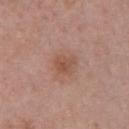Part of a total-body skin-imaging series; this lesion was reviewed on a skin check and was not flagged for biopsy. About 2.5 mm across. A 15 mm crop from a total-body photograph taken for skin-cancer surveillance. The patient is a female aged around 50. Located on the chest. The tile uses white-light illumination.